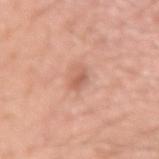Q: Was a biopsy performed?
A: imaged on a skin check; not biopsied
Q: What lighting was used for the tile?
A: white-light
Q: Lesion location?
A: the left upper arm
Q: What did automated image analysis measure?
A: a footprint of about 3 mm², an outline eccentricity of about 0.85 (0 = round, 1 = elongated), and two-axis asymmetry of about 0.25; about 9 CIELAB-L* units darker than the surrounding skin
Q: Who is the patient?
A: male, in their 20s
Q: What is the imaging modality?
A: ~15 mm crop, total-body skin-cancer survey
Q: How large is the lesion?
A: ~2.5 mm (longest diameter)